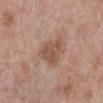anatomic site: the left lower leg; acquisition: ~15 mm crop, total-body skin-cancer survey; subject: female, aged 68–72.